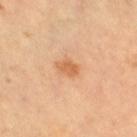Imaged during a routine full-body skin examination; the lesion was not biopsied and no histopathology is available. An algorithmic analysis of the crop reported an outline eccentricity of about 0.75 (0 = round, 1 = elongated) and a shape-asymmetry score of about 0.3 (0 = symmetric). It also reported a mean CIELAB color near L≈62 a*≈24 b*≈40, roughly 10 lightness units darker than nearby skin, and a normalized lesion–skin contrast near 7. The software also gave border irregularity of about 2.5 on a 0–10 scale. The subject is in their 60s. About 2.5 mm across. On the right thigh. This image is a 15 mm lesion crop taken from a total-body photograph. Imaged with cross-polarized lighting.